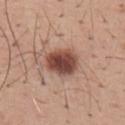Impression:
Imaged during a routine full-body skin examination; the lesion was not biopsied and no histopathology is available.
Acquisition and patient details:
A male subject, aged around 40. The lesion is on the back. Automated image analysis of the tile measured a lesion area of about 10 mm² and an eccentricity of roughly 0.65. The software also gave a border-irregularity rating of about 1.5/10, a color-variation rating of about 4.5/10, and a peripheral color-asymmetry measure near 1.5. A 15 mm crop from a total-body photograph taken for skin-cancer surveillance. Longest diameter approximately 4 mm.